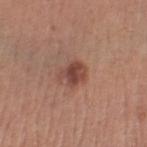notes: catalogued during a skin exam; not biopsied | subject: female, aged 28–32 | site: the right lower leg | lesion diameter: ≈3 mm | illumination: white-light illumination | acquisition: ~15 mm tile from a whole-body skin photo.